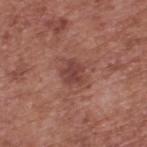follow-up: catalogued during a skin exam; not biopsied | body site: the upper back | illumination: white-light | automated lesion analysis: a border-irregularity rating of about 3/10, a within-lesion color-variation index near 2.5/10, and a peripheral color-asymmetry measure near 1; a lesion-detection confidence of about 100/100 | imaging modality: ~15 mm tile from a whole-body skin photo | subject: male, aged approximately 75 | diameter: about 2.5 mm.